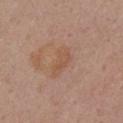automated lesion analysis=a color-variation rating of about 2/10; acquisition=~15 mm crop, total-body skin-cancer survey; body site=the right upper arm; lighting=white-light; patient=female, about 55 years old.